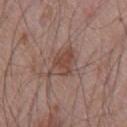biopsy_status: not biopsied; imaged during a skin examination
image:
  source: total-body photography crop
  field_of_view_mm: 15
automated_metrics:
  area_mm2_approx: 8.5
  eccentricity: 0.75
  shape_asymmetry: 0.3
  nevus_likeness_0_100: 50
site: front of the torso
lesion_size:
  long_diameter_mm_approx: 4.5
patient:
  sex: male
  age_approx: 70
lighting: white-light